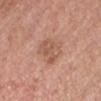Image and clinical context:
Located on the head or neck. A male subject in their 50s. A lesion tile, about 15 mm wide, cut from a 3D total-body photograph. Imaged with white-light lighting. The total-body-photography lesion software estimated roughly 8 lightness units darker than nearby skin and a normalized border contrast of about 5.5. The analysis additionally found a nevus-likeness score of about 0/100 and lesion-presence confidence of about 100/100.